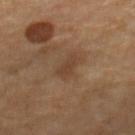<case>
  <biopsy_status>not biopsied; imaged during a skin examination</biopsy_status>
  <patient>
    <sex>female</sex>
    <age_approx>70</age_approx>
  </patient>
  <image>
    <source>total-body photography crop</source>
    <field_of_view_mm>15</field_of_view_mm>
  </image>
  <site>right lower leg</site>
</case>